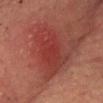Impression: The lesion was photographed on a routine skin check and not biopsied; there is no pathology result. Acquisition and patient details: The tile uses cross-polarized illumination. On the chest. The recorded lesion diameter is about 6.5 mm. Automated tile analysis of the lesion measured an area of roughly 21 mm², a shape eccentricity near 0.75, and two-axis asymmetry of about 0.3. The software also gave a lesion–skin lightness drop of about 6. The analysis additionally found internal color variation of about 3.5 on a 0–10 scale and peripheral color asymmetry of about 1. The subject is a male aged 73–77. A close-up tile cropped from a whole-body skin photograph, about 15 mm across.